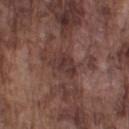Part of a total-body skin-imaging series; this lesion was reviewed on a skin check and was not flagged for biopsy. The lesion is on the left upper arm. A roughly 15 mm field-of-view crop from a total-body skin photograph. The patient is a male about 75 years old. The recorded lesion diameter is about 3.5 mm. Imaged with white-light lighting.